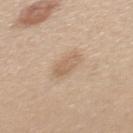lighting: white-light | patient: female, aged around 40 | size: ≈3 mm | image source: ~15 mm tile from a whole-body skin photo | location: the back | image-analysis metrics: a border-irregularity index near 2/10, a within-lesion color-variation index near 3/10, and peripheral color asymmetry of about 1; an automated nevus-likeness rating near 10 out of 100 and lesion-presence confidence of about 100/100.